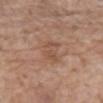| feature | finding |
|---|---|
| biopsy status | imaged on a skin check; not biopsied |
| TBP lesion metrics | a symmetry-axis asymmetry near 0.5; a lesion color around L≈51 a*≈20 b*≈29 in CIELAB, about 7 CIELAB-L* units darker than the surrounding skin, and a normalized lesion–skin contrast near 5; an automated nevus-likeness rating near 0 out of 100 and a detector confidence of about 100 out of 100 that the crop contains a lesion |
| subject | male, roughly 60 years of age |
| imaging modality | ~15 mm tile from a whole-body skin photo |
| anatomic site | the front of the torso |
| lighting | white-light illumination |
| diameter | ~3.5 mm (longest diameter) |